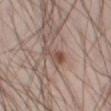{"biopsy_status": "not biopsied; imaged during a skin examination", "patient": {"sex": "male", "age_approx": 45}, "image": {"source": "total-body photography crop", "field_of_view_mm": 15}, "automated_metrics": {"cielab_L": 50, "cielab_a": 18, "cielab_b": 24, "vs_skin_darker_L": 9.0, "vs_skin_contrast_norm": 7.0, "nevus_likeness_0_100": 10, "lesion_detection_confidence_0_100": 100}, "site": "leg", "lesion_size": {"long_diameter_mm_approx": 3.0}}